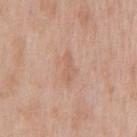Clinical summary: The lesion is on the chest. A 15 mm close-up extracted from a 3D total-body photography capture. A male patient roughly 65 years of age.